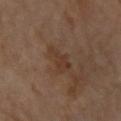Captured during whole-body skin photography for melanoma surveillance; the lesion was not biopsied.
About 4 mm across.
The subject is a female aged around 70.
The total-body-photography lesion software estimated a lesion color around L≈36 a*≈17 b*≈27 in CIELAB and roughly 6 lightness units darker than nearby skin. And it measured a border-irregularity rating of about 5/10, a within-lesion color-variation index near 2/10, and a peripheral color-asymmetry measure near 0.5.
Imaged with cross-polarized lighting.
From the left forearm.
A roughly 15 mm field-of-view crop from a total-body skin photograph.